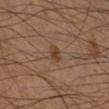Assessment:
No biopsy was performed on this lesion — it was imaged during a full skin examination and was not determined to be concerning.
Acquisition and patient details:
The lesion is on the right lower leg. A male subject, approximately 55 years of age. The tile uses cross-polarized illumination. The lesion's longest dimension is about 2 mm. This image is a 15 mm lesion crop taken from a total-body photograph.